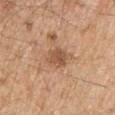Case summary:
– biopsy status: total-body-photography surveillance lesion; no biopsy
– anatomic site: the upper back
– patient: male, approximately 70 years of age
– lighting: white-light
– imaging modality: ~15 mm crop, total-body skin-cancer survey
– image-analysis metrics: a footprint of about 5.5 mm², an outline eccentricity of about 0.6 (0 = round, 1 = elongated), and two-axis asymmetry of about 0.25; an average lesion color of about L≈53 a*≈21 b*≈33 (CIELAB), a lesion–skin lightness drop of about 10, and a lesion-to-skin contrast of about 7 (normalized; higher = more distinct); an automated nevus-likeness rating near 20 out of 100 and a detector confidence of about 100 out of 100 that the crop contains a lesion
– size: about 3 mm